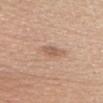Captured under white-light illumination. Cropped from a whole-body photographic skin survey; the tile spans about 15 mm. A female subject roughly 40 years of age. Measured at roughly 3 mm in maximum diameter. On the head or neck.